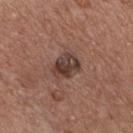An algorithmic analysis of the crop reported a footprint of about 7.5 mm², an outline eccentricity of about 0.6 (0 = round, 1 = elongated), and a shape-asymmetry score of about 0.2 (0 = symmetric). And it measured a lesion color around L≈39 a*≈18 b*≈22 in CIELAB, about 11 CIELAB-L* units darker than the surrounding skin, and a lesion-to-skin contrast of about 9.5 (normalized; higher = more distinct).
A 15 mm close-up extracted from a 3D total-body photography capture.
The lesion is on the chest.
This is a white-light tile.
The subject is a male approximately 55 years of age.
Longest diameter approximately 3.5 mm.